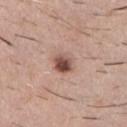The lesion was photographed on a routine skin check and not biopsied; there is no pathology result. From the chest. Captured under white-light illumination. A region of skin cropped from a whole-body photographic capture, roughly 15 mm wide. A male patient, approximately 40 years of age. The recorded lesion diameter is about 2.5 mm.